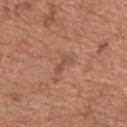This lesion was catalogued during total-body skin photography and was not selected for biopsy.
On the back.
A lesion tile, about 15 mm wide, cut from a 3D total-body photograph.
Longest diameter approximately 2.5 mm.
A female patient, aged approximately 60.
The lesion-visualizer software estimated a classifier nevus-likeness of about 0/100.
Captured under white-light illumination.